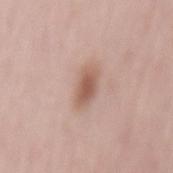follow-up = imaged on a skin check; not biopsied
lesion size = ~4 mm (longest diameter)
TBP lesion metrics = a border-irregularity rating of about 2/10, internal color variation of about 3 on a 0–10 scale, and radial color variation of about 1
body site = the mid back
patient = female, in their mid- to late 60s
image source = ~15 mm crop, total-body skin-cancer survey
lighting = white-light